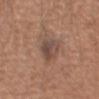Clinical impression:
Recorded during total-body skin imaging; not selected for excision or biopsy.
Background:
The lesion is located on the arm. This image is a 15 mm lesion crop taken from a total-body photograph. Measured at roughly 3 mm in maximum diameter. The patient is a male approximately 50 years of age.